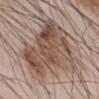Impression:
The lesion was tiled from a total-body skin photograph and was not biopsied.
Context:
The tile uses white-light illumination. An algorithmic analysis of the crop reported an outline eccentricity of about 0.8 (0 = round, 1 = elongated) and a shape-asymmetry score of about 0.35 (0 = symmetric). It also reported an average lesion color of about L≈51 a*≈16 b*≈24 (CIELAB), a lesion–skin lightness drop of about 11, and a normalized border contrast of about 8. The software also gave a border-irregularity index near 5/10, a within-lesion color-variation index near 7.5/10, and peripheral color asymmetry of about 2.5. It also reported an automated nevus-likeness rating near 15 out of 100 and lesion-presence confidence of about 65/100. Located on the abdomen. A lesion tile, about 15 mm wide, cut from a 3D total-body photograph. A male patient aged 53–57.